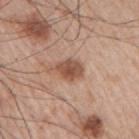follow-up: catalogued during a skin exam; not biopsied.